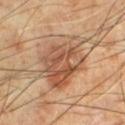Impression:
No biopsy was performed on this lesion — it was imaged during a full skin examination and was not determined to be concerning.
Clinical summary:
The subject is a male aged around 70. This is a cross-polarized tile. From the left lower leg. A lesion tile, about 15 mm wide, cut from a 3D total-body photograph. Automated tile analysis of the lesion measured an area of roughly 24 mm² and a symmetry-axis asymmetry near 0.25. The analysis additionally found a mean CIELAB color near L≈52 a*≈21 b*≈32 and a normalized border contrast of about 7.5. The analysis additionally found border irregularity of about 3 on a 0–10 scale, internal color variation of about 7 on a 0–10 scale, and radial color variation of about 2.5. Approximately 6 mm at its widest.